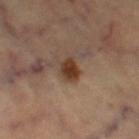Imaged during a routine full-body skin examination; the lesion was not biopsied and no histopathology is available. A female subject about 65 years old. This image is a 15 mm lesion crop taken from a total-body photograph. Located on the right thigh.